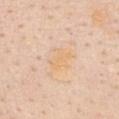{
  "biopsy_status": "not biopsied; imaged during a skin examination",
  "image": {
    "source": "total-body photography crop",
    "field_of_view_mm": 15
  },
  "site": "chest",
  "lighting": "white-light",
  "patient": {
    "sex": "female",
    "age_approx": 60
  }
}